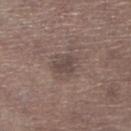{
  "biopsy_status": "not biopsied; imaged during a skin examination",
  "image": {
    "source": "total-body photography crop",
    "field_of_view_mm": 15
  },
  "lesion_size": {
    "long_diameter_mm_approx": 2.5
  },
  "patient": {
    "sex": "male",
    "age_approx": 70
  },
  "automated_metrics": {
    "border_irregularity_0_10": 4.0,
    "color_variation_0_10": 1.0,
    "lesion_detection_confidence_0_100": 100
  },
  "site": "leg"
}